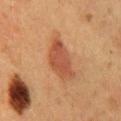Clinical impression:
No biopsy was performed on this lesion — it was imaged during a full skin examination and was not determined to be concerning.
Context:
Longest diameter approximately 5.5 mm. Cropped from a whole-body photographic skin survey; the tile spans about 15 mm. A female subject, in their 50s. The tile uses cross-polarized illumination. The lesion is on the lower back.